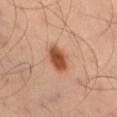Imaged during a routine full-body skin examination; the lesion was not biopsied and no histopathology is available. Imaged with cross-polarized lighting. Longest diameter approximately 4 mm. The lesion is on the left thigh. A 15 mm close-up extracted from a 3D total-body photography capture. Automated tile analysis of the lesion measured a footprint of about 7 mm². And it measured an average lesion color of about L≈51 a*≈25 b*≈34 (CIELAB), roughly 15 lightness units darker than nearby skin, and a normalized lesion–skin contrast near 10.5. The software also gave border irregularity of about 1.5 on a 0–10 scale. It also reported a detector confidence of about 100 out of 100 that the crop contains a lesion. A male patient, in their 50s.